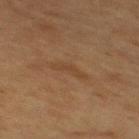Captured during whole-body skin photography for melanoma surveillance; the lesion was not biopsied.
The lesion is on the back.
The tile uses cross-polarized illumination.
A female patient, aged 38–42.
About 3.5 mm across.
Automated image analysis of the tile measured a border-irregularity rating of about 6.5/10, a color-variation rating of about 0/10, and a peripheral color-asymmetry measure near 0. It also reported a classifier nevus-likeness of about 0/100 and a lesion-detection confidence of about 100/100.
A roughly 15 mm field-of-view crop from a total-body skin photograph.